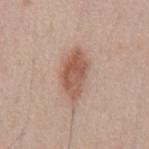follow-up: total-body-photography surveillance lesion; no biopsy
image-analysis metrics: a lesion color around L≈56 a*≈21 b*≈28 in CIELAB, a lesion–skin lightness drop of about 12, and a normalized lesion–skin contrast near 8; an automated nevus-likeness rating near 85 out of 100 and a lesion-detection confidence of about 100/100
image: ~15 mm crop, total-body skin-cancer survey
subject: male, aged 48 to 52
location: the mid back